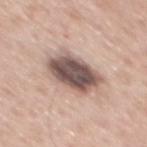Q: Is there a histopathology result?
A: no biopsy performed (imaged during a skin exam)